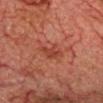The lesion was photographed on a routine skin check and not biopsied; there is no pathology result.
On the head or neck.
Automated tile analysis of the lesion measured an area of roughly 5.5 mm², a shape eccentricity near 0.85, and two-axis asymmetry of about 0.3. It also reported a lesion color around L≈36 a*≈26 b*≈27 in CIELAB, a lesion–skin lightness drop of about 6, and a normalized lesion–skin contrast near 6. It also reported a border-irregularity rating of about 3/10 and peripheral color asymmetry of about 1.5.
Imaged with cross-polarized lighting.
A male subject in their mid- to late 70s.
Cropped from a whole-body photographic skin survey; the tile spans about 15 mm.
The recorded lesion diameter is about 3.5 mm.